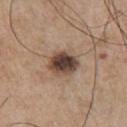Captured during whole-body skin photography for melanoma surveillance; the lesion was not biopsied. A 15 mm crop from a total-body photograph taken for skin-cancer surveillance. The tile uses white-light illumination. On the front of the torso. A male patient, in their mid- to late 60s. The lesion-visualizer software estimated a lesion area of about 10 mm², an outline eccentricity of about 0.6 (0 = round, 1 = elongated), and a shape-asymmetry score of about 0.15 (0 = symmetric). It also reported an average lesion color of about L≈44 a*≈16 b*≈24 (CIELAB) and a lesion–skin lightness drop of about 17. Approximately 4 mm at its widest.